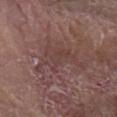The lesion was tiled from a total-body skin photograph and was not biopsied.
Automated image analysis of the tile measured roughly 6 lightness units darker than nearby skin and a lesion-to-skin contrast of about 5 (normalized; higher = more distinct). And it measured a border-irregularity index near 9.5/10, internal color variation of about 3 on a 0–10 scale, and peripheral color asymmetry of about 1. And it measured a classifier nevus-likeness of about 0/100 and a lesion-detection confidence of about 80/100.
On the right arm.
The patient is a male aged 78–82.
Cropped from a total-body skin-imaging series; the visible field is about 15 mm.
Approximately 7.5 mm at its widest.
This is a white-light tile.